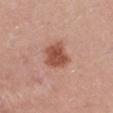– biopsy status — catalogued during a skin exam; not biopsied
– patient — male, about 40 years old
– lesion diameter — ~3.5 mm (longest diameter)
– imaging modality — 15 mm crop, total-body photography
– tile lighting — white-light
– anatomic site — the left upper arm